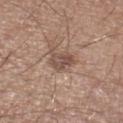Notes:
– notes — total-body-photography surveillance lesion; no biopsy
– lighting — white-light
– automated lesion analysis — an average lesion color of about L≈49 a*≈18 b*≈24 (CIELAB) and a normalized lesion–skin contrast near 7.5; a border-irregularity rating of about 3/10 and peripheral color asymmetry of about 2; a nevus-likeness score of about 15/100
– acquisition — ~15 mm crop, total-body skin-cancer survey
– diameter — about 3 mm
– subject — male, aged 63 to 67
– anatomic site — the left lower leg